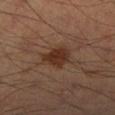The lesion was tiled from a total-body skin photograph and was not biopsied. Imaged with cross-polarized lighting. Cropped from a whole-body photographic skin survey; the tile spans about 15 mm. The lesion is on the right lower leg. Measured at roughly 3.5 mm in maximum diameter. A male patient in their mid- to late 50s.